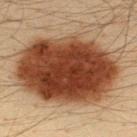notes: total-body-photography surveillance lesion; no biopsy
patient: male, aged around 35
illumination: cross-polarized
automated metrics: an outline eccentricity of about 0.7 (0 = round, 1 = elongated) and two-axis asymmetry of about 0.2; a border-irregularity index near 2.5/10 and peripheral color asymmetry of about 3; a classifier nevus-likeness of about 100/100
acquisition: ~15 mm crop, total-body skin-cancer survey
anatomic site: the upper back